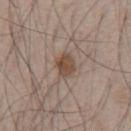Impression: Part of a total-body skin-imaging series; this lesion was reviewed on a skin check and was not flagged for biopsy.